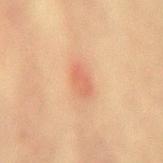{
  "biopsy_status": "not biopsied; imaged during a skin examination",
  "lesion_size": {
    "long_diameter_mm_approx": 3.0
  },
  "site": "lower back",
  "image": {
    "source": "total-body photography crop",
    "field_of_view_mm": 15
  },
  "lighting": "cross-polarized",
  "automated_metrics": {
    "area_mm2_approx": 4.5,
    "border_irregularity_0_10": 2.5,
    "peripheral_color_asymmetry": 1.5
  },
  "patient": {
    "sex": "female",
    "age_approx": 65
  }
}